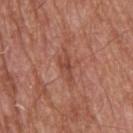follow-up = no biopsy performed (imaged during a skin exam); location = the upper back; subject = male, aged around 65; acquisition = ~15 mm crop, total-body skin-cancer survey.